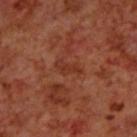workup: catalogued during a skin exam; not biopsied | acquisition: ~15 mm tile from a whole-body skin photo | lighting: cross-polarized illumination | subject: male, in their 70s | automated metrics: a footprint of about 3.5 mm², an eccentricity of roughly 0.9, and a symmetry-axis asymmetry near 0.5; a peripheral color-asymmetry measure near 0 | size: about 3 mm | body site: the upper back.